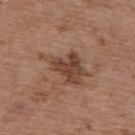{"biopsy_status": "not biopsied; imaged during a skin examination", "site": "upper back", "image": {"source": "total-body photography crop", "field_of_view_mm": 15}, "patient": {"sex": "female", "age_approx": 65}, "lesion_size": {"long_diameter_mm_approx": 6.0}}